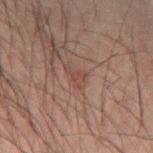<record>
<biopsy_status>not biopsied; imaged during a skin examination</biopsy_status>
<patient>
  <sex>male</sex>
  <age_approx>40</age_approx>
</patient>
<site>right forearm</site>
<lesion_size>
  <long_diameter_mm_approx>2.5</long_diameter_mm_approx>
</lesion_size>
<automated_metrics>
  <cielab_L>36</cielab_L>
  <cielab_a>16</cielab_a>
  <cielab_b>21</cielab_b>
  <vs_skin_darker_L>5.0</vs_skin_darker_L>
  <vs_skin_contrast_norm>5.0</vs_skin_contrast_norm>
</automated_metrics>
<image>
  <source>total-body photography crop</source>
  <field_of_view_mm>15</field_of_view_mm>
</image>
</record>